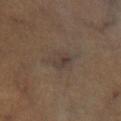{"biopsy_status": "not biopsied; imaged during a skin examination", "image": {"source": "total-body photography crop", "field_of_view_mm": 15}, "site": "left lower leg", "automated_metrics": {"eccentricity": 0.75, "shape_asymmetry": 0.3, "cielab_L": 37, "cielab_a": 11, "cielab_b": 20, "vs_skin_darker_L": 6.0, "vs_skin_contrast_norm": 6.0, "border_irregularity_0_10": 3.0, "color_variation_0_10": 5.0, "peripheral_color_asymmetry": 2.0, "nevus_likeness_0_100": 0}, "patient": {"sex": "male", "age_approx": 50}}